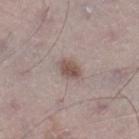Q: Was this lesion biopsied?
A: total-body-photography surveillance lesion; no biopsy
Q: Illumination type?
A: white-light illumination
Q: Who is the patient?
A: male, roughly 70 years of age
Q: What did automated image analysis measure?
A: a lesion color around L≈51 a*≈16 b*≈21 in CIELAB, about 11 CIELAB-L* units darker than the surrounding skin, and a lesion-to-skin contrast of about 8 (normalized; higher = more distinct); a nevus-likeness score of about 85/100 and a detector confidence of about 100 out of 100 that the crop contains a lesion
Q: What kind of image is this?
A: ~15 mm tile from a whole-body skin photo
Q: How large is the lesion?
A: ~2.5 mm (longest diameter)
Q: What is the anatomic site?
A: the right lower leg